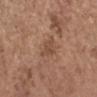The lesion was tiled from a total-body skin photograph and was not biopsied.
Imaged with white-light lighting.
A 15 mm close-up extracted from a 3D total-body photography capture.
On the left forearm.
A female patient, aged 63 to 67.